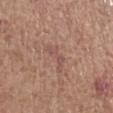No biopsy was performed on this lesion — it was imaged during a full skin examination and was not determined to be concerning. This image is a 15 mm lesion crop taken from a total-body photograph. Imaged with white-light lighting. A female subject, about 65 years old. The lesion is located on the arm.